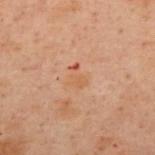Q: Was this lesion biopsied?
A: total-body-photography surveillance lesion; no biopsy
Q: What kind of image is this?
A: ~15 mm tile from a whole-body skin photo
Q: Lesion size?
A: about 2.5 mm
Q: What are the patient's age and sex?
A: female, approximately 55 years of age
Q: How was the tile lit?
A: cross-polarized illumination
Q: What did automated image analysis measure?
A: a lesion color around L≈49 a*≈20 b*≈31 in CIELAB, a lesion–skin lightness drop of about 5, and a normalized border contrast of about 5; border irregularity of about 4.5 on a 0–10 scale, a within-lesion color-variation index near 1/10, and a peripheral color-asymmetry measure near 0.5; a nevus-likeness score of about 0/100 and a lesion-detection confidence of about 100/100
Q: What is the anatomic site?
A: the upper back